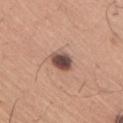On the right upper arm.
About 3 mm across.
A male patient aged 43 to 47.
A roughly 15 mm field-of-view crop from a total-body skin photograph.